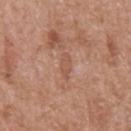Part of a total-body skin-imaging series; this lesion was reviewed on a skin check and was not flagged for biopsy. Captured under white-light illumination. A male patient about 65 years old. Longest diameter approximately 3 mm. On the abdomen. A close-up tile cropped from a whole-body skin photograph, about 15 mm across.